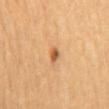biopsy_status: not biopsied; imaged during a skin examination
lesion_size:
  long_diameter_mm_approx: 2.0
image:
  source: total-body photography crop
  field_of_view_mm: 15
site: mid back
patient:
  sex: female
  age_approx: 65